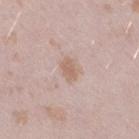workup: no biopsy performed (imaged during a skin exam); diameter: ≈2.5 mm; body site: the leg; patient: female, aged 23 to 27; imaging modality: 15 mm crop, total-body photography.